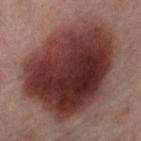Assessment: The lesion was photographed on a routine skin check and not biopsied; there is no pathology result. Image and clinical context: Captured under cross-polarized illumination. About 11.5 mm across. A roughly 15 mm field-of-view crop from a total-body skin photograph. The subject is a female about 55 years old. Located on the right thigh.